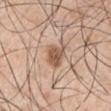notes: no biopsy performed (imaged during a skin exam)
acquisition: ~15 mm tile from a whole-body skin photo
subject: male, aged approximately 50
image-analysis metrics: a lesion area of about 6 mm² and two-axis asymmetry of about 0.3; an average lesion color of about L≈56 a*≈20 b*≈30 (CIELAB), roughly 12 lightness units darker than nearby skin, and a lesion-to-skin contrast of about 8.5 (normalized; higher = more distinct)
tile lighting: white-light
diameter: ≈3.5 mm
site: the left upper arm